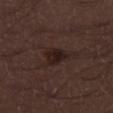The lesion was photographed on a routine skin check and not biopsied; there is no pathology result.
Approximately 3 mm at its widest.
A male patient about 30 years old.
On the right thigh.
A region of skin cropped from a whole-body photographic capture, roughly 15 mm wide.